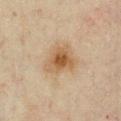The lesion was photographed on a routine skin check and not biopsied; there is no pathology result. A region of skin cropped from a whole-body photographic capture, roughly 15 mm wide. Located on the front of the torso. Approximately 4 mm at its widest. The total-body-photography lesion software estimated a lesion area of about 9.5 mm², an outline eccentricity of about 0.6 (0 = round, 1 = elongated), and a symmetry-axis asymmetry near 0.35. And it measured a nevus-likeness score of about 85/100. A male subject, aged around 45. Imaged with cross-polarized lighting.